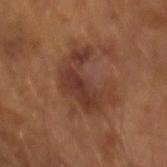Clinical impression:
No biopsy was performed on this lesion — it was imaged during a full skin examination and was not determined to be concerning.
Context:
An algorithmic analysis of the crop reported an average lesion color of about L≈35 a*≈21 b*≈26 (CIELAB) and a normalized lesion–skin contrast near 8. And it measured an automated nevus-likeness rating near 10 out of 100 and a lesion-detection confidence of about 100/100. A 15 mm crop from a total-body photograph taken for skin-cancer surveillance. Imaged with cross-polarized lighting. A male patient aged around 65.